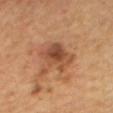Imaged during a routine full-body skin examination; the lesion was not biopsied and no histopathology is available.
The lesion's longest dimension is about 4 mm.
A region of skin cropped from a whole-body photographic capture, roughly 15 mm wide.
The tile uses cross-polarized illumination.
Located on the back.
The subject is female.